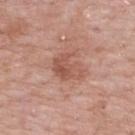No biopsy was performed on this lesion — it was imaged during a full skin examination and was not determined to be concerning.
A close-up tile cropped from a whole-body skin photograph, about 15 mm across.
The subject is a male aged approximately 55.
Measured at roughly 3.5 mm in maximum diameter.
From the back.
The total-body-photography lesion software estimated a lesion–skin lightness drop of about 8 and a normalized border contrast of about 6. And it measured a border-irregularity rating of about 4.5/10 and a within-lesion color-variation index near 4.5/10.